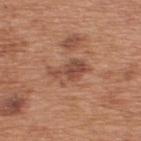follow-up: catalogued during a skin exam; not biopsied | automated lesion analysis: a lesion area of about 6 mm² and an outline eccentricity of about 0.9 (0 = round, 1 = elongated); a nevus-likeness score of about 0/100 and a lesion-detection confidence of about 100/100 | body site: the upper back | diameter: ≈4 mm | acquisition: total-body-photography crop, ~15 mm field of view | lighting: white-light | patient: male, approximately 65 years of age.